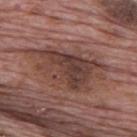This lesion was catalogued during total-body skin photography and was not selected for biopsy. Cropped from a total-body skin-imaging series; the visible field is about 15 mm. A male subject, in their 70s. The lesion's longest dimension is about 9 mm. From the mid back. Automated tile analysis of the lesion measured a mean CIELAB color near L≈40 a*≈20 b*≈22, roughly 10 lightness units darker than nearby skin, and a normalized lesion–skin contrast near 8. Imaged with white-light lighting.